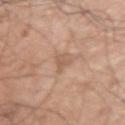Clinical impression:
No biopsy was performed on this lesion — it was imaged during a full skin examination and was not determined to be concerning.
Image and clinical context:
The total-body-photography lesion software estimated an area of roughly 3 mm², a shape eccentricity near 0.8, and two-axis asymmetry of about 0.45. It also reported an average lesion color of about L≈58 a*≈19 b*≈30 (CIELAB), roughly 7 lightness units darker than nearby skin, and a normalized lesion–skin contrast near 5. The analysis additionally found a nevus-likeness score of about 0/100 and a lesion-detection confidence of about 100/100. The patient is a male approximately 65 years of age. Imaged with white-light lighting. A lesion tile, about 15 mm wide, cut from a 3D total-body photograph. On the left upper arm.